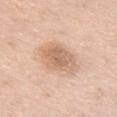This lesion was catalogued during total-body skin photography and was not selected for biopsy.
An algorithmic analysis of the crop reported a mean CIELAB color near L≈66 a*≈19 b*≈31 and roughly 11 lightness units darker than nearby skin. And it measured a border-irregularity rating of about 2/10, a color-variation rating of about 4/10, and a peripheral color-asymmetry measure near 1.5.
On the chest.
A female subject, in their mid-60s.
A lesion tile, about 15 mm wide, cut from a 3D total-body photograph.
Longest diameter approximately 6 mm.
Imaged with white-light lighting.